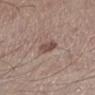Captured during whole-body skin photography for melanoma surveillance; the lesion was not biopsied.
Imaged with white-light lighting.
About 2.5 mm across.
From the left lower leg.
A region of skin cropped from a whole-body photographic capture, roughly 15 mm wide.
The total-body-photography lesion software estimated border irregularity of about 2 on a 0–10 scale and internal color variation of about 2 on a 0–10 scale.
A male subject, in their mid- to late 50s.